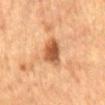Part of a total-body skin-imaging series; this lesion was reviewed on a skin check and was not flagged for biopsy. The lesion's longest dimension is about 4.5 mm. A male patient about 85 years old or older. An algorithmic analysis of the crop reported an average lesion color of about L≈48 a*≈22 b*≈35 (CIELAB), roughly 14 lightness units darker than nearby skin, and a normalized border contrast of about 10. The analysis additionally found a border-irregularity rating of about 2.5/10, internal color variation of about 4 on a 0–10 scale, and a peripheral color-asymmetry measure near 1. From the back. Captured under cross-polarized illumination. This image is a 15 mm lesion crop taken from a total-body photograph.